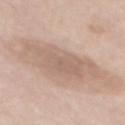{"biopsy_status": "not biopsied; imaged during a skin examination"}